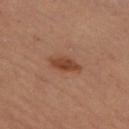biopsy_status: not biopsied; imaged during a skin examination
image:
  source: total-body photography crop
  field_of_view_mm: 15
lesion_size:
  long_diameter_mm_approx: 4.0
site: left thigh
automated_metrics:
  shape_asymmetry: 0.2
  border_irregularity_0_10: 2.5
  color_variation_0_10: 2.0
  nevus_likeness_0_100: 95
  lesion_detection_confidence_0_100: 100
lighting: cross-polarized
patient:
  sex: female
  age_approx: 50